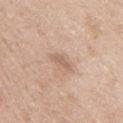Impression:
The lesion was photographed on a routine skin check and not biopsied; there is no pathology result.
Background:
The lesion-visualizer software estimated a border-irregularity index near 4/10, a color-variation rating of about 0/10, and a peripheral color-asymmetry measure near 0. It also reported a nevus-likeness score of about 0/100 and a detector confidence of about 100 out of 100 that the crop contains a lesion. This image is a 15 mm lesion crop taken from a total-body photograph. The subject is a male aged 63 to 67. Measured at roughly 3 mm in maximum diameter. Located on the mid back.